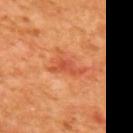image: ~15 mm crop, total-body skin-cancer survey | image-analysis metrics: a lesion area of about 7 mm² and an outline eccentricity of about 0.8 (0 = round, 1 = elongated); an average lesion color of about L≈55 a*≈35 b*≈41 (CIELAB), roughly 9 lightness units darker than nearby skin, and a normalized lesion–skin contrast near 6 | body site: the back | patient: female, aged 38–42 | illumination: cross-polarized | lesion diameter: ≈4.5 mm.